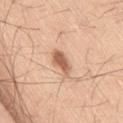Part of a total-body skin-imaging series; this lesion was reviewed on a skin check and was not flagged for biopsy. Imaged with white-light lighting. A male subject roughly 60 years of age. The lesion is located on the right thigh. Approximately 4 mm at its widest. A roughly 15 mm field-of-view crop from a total-body skin photograph.